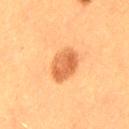A 15 mm close-up tile from a total-body photography series done for melanoma screening. A female subject roughly 40 years of age. The total-body-photography lesion software estimated an area of roughly 10 mm². It also reported an average lesion color of about L≈53 a*≈25 b*≈38 (CIELAB), a lesion–skin lightness drop of about 12, and a lesion-to-skin contrast of about 8 (normalized; higher = more distinct). The software also gave radial color variation of about 1. Located on the left thigh.